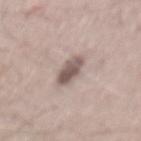Recorded during total-body skin imaging; not selected for excision or biopsy. Captured under white-light illumination. The lesion's longest dimension is about 3.5 mm. A 15 mm crop from a total-body photograph taken for skin-cancer surveillance. The total-body-photography lesion software estimated a within-lesion color-variation index near 4.5/10. From the mid back. A male patient, aged around 70.